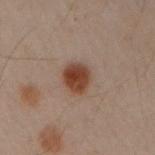notes — imaged on a skin check; not biopsied | lighting — cross-polarized illumination | image — ~15 mm crop, total-body skin-cancer survey | subject — male, aged around 50 | site — the left arm | TBP lesion metrics — a footprint of about 7.5 mm², an outline eccentricity of about 0.7 (0 = round, 1 = elongated), and a shape-asymmetry score of about 0.15 (0 = symmetric); a border-irregularity rating of about 1.5/10, internal color variation of about 3.5 on a 0–10 scale, and a peripheral color-asymmetry measure near 1.